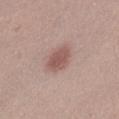Findings:
– body site · the left thigh
– subject · female, about 20 years old
– acquisition · ~15 mm tile from a whole-body skin photo
– tile lighting · white-light
– size · ≈3 mm